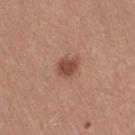The lesion's longest dimension is about 3 mm. A region of skin cropped from a whole-body photographic capture, roughly 15 mm wide. The patient is a female in their 50s. The lesion is on the right thigh. Automated image analysis of the tile measured a footprint of about 6 mm², an eccentricity of roughly 0.55, and a shape-asymmetry score of about 0.2 (0 = symmetric). The analysis additionally found a border-irregularity index near 2/10, a within-lesion color-variation index near 3/10, and peripheral color asymmetry of about 1. The software also gave a classifier nevus-likeness of about 95/100 and a detector confidence of about 100 out of 100 that the crop contains a lesion. The tile uses white-light illumination.